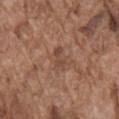{"biopsy_status": "not biopsied; imaged during a skin examination", "lesion_size": {"long_diameter_mm_approx": 3.5}, "lighting": "white-light", "site": "chest", "patient": {"sex": "male", "age_approx": 75}, "image": {"source": "total-body photography crop", "field_of_view_mm": 15}}